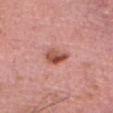The lesion was photographed on a routine skin check and not biopsied; there is no pathology result. From the head or neck. Automated tile analysis of the lesion measured a mean CIELAB color near L≈53 a*≈28 b*≈29, a lesion–skin lightness drop of about 13, and a normalized lesion–skin contrast near 9. The analysis additionally found a border-irregularity rating of about 3/10, internal color variation of about 8.5 on a 0–10 scale, and peripheral color asymmetry of about 3.5. A male patient about 80 years old. A close-up tile cropped from a whole-body skin photograph, about 15 mm across.